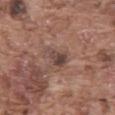biopsy status: imaged on a skin check; not biopsied
lighting: white-light illumination
automated metrics: border irregularity of about 3.5 on a 0–10 scale, a color-variation rating of about 5.5/10, and radial color variation of about 2; an automated nevus-likeness rating near 0 out of 100 and a lesion-detection confidence of about 100/100
image source: 15 mm crop, total-body photography
location: the abdomen
diameter: ≈3.5 mm
subject: male, aged 73 to 77